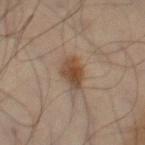Assessment: The lesion was photographed on a routine skin check and not biopsied; there is no pathology result. Background: A male subject, roughly 50 years of age. This image is a 15 mm lesion crop taken from a total-body photograph. Captured under cross-polarized illumination. An algorithmic analysis of the crop reported a lesion color around L≈45 a*≈17 b*≈29 in CIELAB, about 11 CIELAB-L* units darker than the surrounding skin, and a normalized lesion–skin contrast near 9. The lesion is on the lower back.